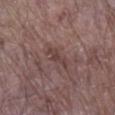No biopsy was performed on this lesion — it was imaged during a full skin examination and was not determined to be concerning.
A male patient, aged 78–82.
The lesion's longest dimension is about 3.5 mm.
From the right lower leg.
A roughly 15 mm field-of-view crop from a total-body skin photograph.